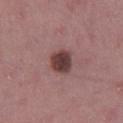workup — total-body-photography surveillance lesion; no biopsy | subject — female, aged approximately 45 | anatomic site — the right thigh | illumination — white-light | imaging modality — ~15 mm tile from a whole-body skin photo | diameter — ≈3 mm.